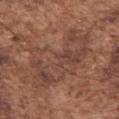Q: Was this lesion biopsied?
A: imaged on a skin check; not biopsied
Q: What are the patient's age and sex?
A: male, in their mid- to late 70s
Q: Lesion location?
A: the left upper arm
Q: How was this image acquired?
A: ~15 mm crop, total-body skin-cancer survey
Q: Lesion size?
A: ≈9.5 mm
Q: What did automated image analysis measure?
A: an eccentricity of roughly 0.85 and two-axis asymmetry of about 0.45; a classifier nevus-likeness of about 0/100 and a detector confidence of about 60 out of 100 that the crop contains a lesion